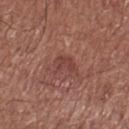A male subject, aged around 70.
The lesion is located on the right lower leg.
A region of skin cropped from a whole-body photographic capture, roughly 15 mm wide.